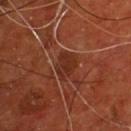biopsy status = imaged on a skin check; not biopsied
lesion size = about 3.5 mm
acquisition = ~15 mm crop, total-body skin-cancer survey
patient = male, roughly 55 years of age
site = the chest
automated metrics = an area of roughly 6 mm², an eccentricity of roughly 0.7, and two-axis asymmetry of about 0.25; an average lesion color of about L≈28 a*≈24 b*≈28 (CIELAB); a border-irregularity rating of about 2.5/10, internal color variation of about 2 on a 0–10 scale, and peripheral color asymmetry of about 1; an automated nevus-likeness rating near 0 out of 100 and lesion-presence confidence of about 90/100
lighting = cross-polarized